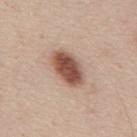anatomic site: the back
subject: male, aged 43 to 47
automated lesion analysis: a lesion color around L≈51 a*≈21 b*≈27 in CIELAB, roughly 17 lightness units darker than nearby skin, and a lesion-to-skin contrast of about 11.5 (normalized; higher = more distinct); an automated nevus-likeness rating near 100 out of 100
image source: ~15 mm crop, total-body skin-cancer survey
illumination: white-light illumination
lesion size: ≈5 mm